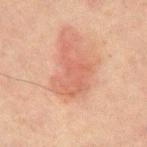Notes:
– biopsy status · no biopsy performed (imaged during a skin exam)